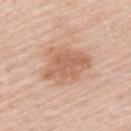  biopsy_status: not biopsied; imaged during a skin examination
  image:
    source: total-body photography crop
    field_of_view_mm: 15
  site: upper back
  patient:
    sex: male
    age_approx: 60